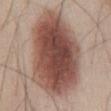A roughly 15 mm field-of-view crop from a total-body skin photograph.
A male patient, in their mid-40s.
The tile uses white-light illumination.
An algorithmic analysis of the crop reported a footprint of about 65 mm², an eccentricity of roughly 0.85, and a symmetry-axis asymmetry near 0.15. It also reported a lesion color around L≈49 a*≈20 b*≈24 in CIELAB. And it measured an automated nevus-likeness rating near 100 out of 100 and lesion-presence confidence of about 100/100.
The lesion's longest dimension is about 12 mm.
From the chest.